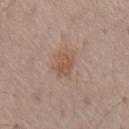Impression: Captured during whole-body skin photography for melanoma surveillance; the lesion was not biopsied. Acquisition and patient details: The lesion is on the chest. A close-up tile cropped from a whole-body skin photograph, about 15 mm across. This is a white-light tile. Automated tile analysis of the lesion measured a mean CIELAB color near L≈54 a*≈19 b*≈28, a lesion–skin lightness drop of about 7, and a normalized lesion–skin contrast near 6. It also reported a border-irregularity rating of about 3/10, a color-variation rating of about 3/10, and radial color variation of about 1. And it measured an automated nevus-likeness rating near 70 out of 100 and a detector confidence of about 100 out of 100 that the crop contains a lesion. About 3.5 mm across. A male subject, aged 63 to 67.